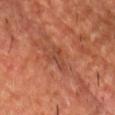Impression:
Imaged during a routine full-body skin examination; the lesion was not biopsied and no histopathology is available.
Acquisition and patient details:
A male patient, aged approximately 60. A lesion tile, about 15 mm wide, cut from a 3D total-body photograph. On the chest.